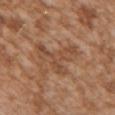biopsy status: catalogued during a skin exam; not biopsied | acquisition: total-body-photography crop, ~15 mm field of view | tile lighting: white-light illumination | patient: female, approximately 30 years of age | automated metrics: an area of roughly 12 mm² and a symmetry-axis asymmetry near 0.75; a lesion color around L≈48 a*≈20 b*≈32 in CIELAB, a lesion–skin lightness drop of about 7, and a lesion-to-skin contrast of about 5.5 (normalized; higher = more distinct); a border-irregularity index near 9.5/10 | size: ≈5.5 mm | location: the chest.